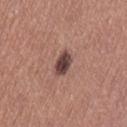notes=imaged on a skin check; not biopsied
automated metrics=a classifier nevus-likeness of about 65/100
anatomic site=the lower back
acquisition=~15 mm crop, total-body skin-cancer survey
tile lighting=white-light
patient=female, aged 48–52
diameter=~3 mm (longest diameter)